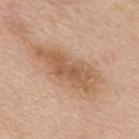<lesion>
  <biopsy_status>not biopsied; imaged during a skin examination</biopsy_status>
  <patient>
    <sex>male</sex>
    <age_approx>50</age_approx>
  </patient>
  <site>mid back</site>
  <image>
    <source>total-body photography crop</source>
    <field_of_view_mm>15</field_of_view_mm>
  </image>
</lesion>